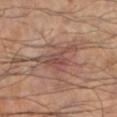Imaged during a routine full-body skin examination; the lesion was not biopsied and no histopathology is available. On the leg. A male patient approximately 60 years of age. Automated image analysis of the tile measured an area of roughly 14 mm², an outline eccentricity of about 0.8 (0 = round, 1 = elongated), and a shape-asymmetry score of about 0.4 (0 = symmetric). And it measured a lesion color around L≈47 a*≈20 b*≈24 in CIELAB, a lesion–skin lightness drop of about 8, and a lesion-to-skin contrast of about 7 (normalized; higher = more distinct). Cropped from a whole-body photographic skin survey; the tile spans about 15 mm. The recorded lesion diameter is about 6.5 mm. Captured under cross-polarized illumination.